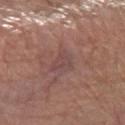biopsy status = no biopsy performed (imaged during a skin exam) | tile lighting = white-light illumination | image source = ~15 mm crop, total-body skin-cancer survey | site = the arm | patient = female, roughly 55 years of age | automated metrics = a lesion area of about 5 mm² and an eccentricity of roughly 0.8; a nevus-likeness score of about 0/100 and a lesion-detection confidence of about 80/100.